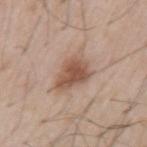• follow-up: no biopsy performed (imaged during a skin exam)